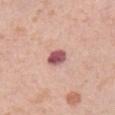Clinical impression:
Captured during whole-body skin photography for melanoma surveillance; the lesion was not biopsied.
Acquisition and patient details:
The tile uses white-light illumination. A 15 mm close-up extracted from a 3D total-body photography capture. A female patient, aged around 40. Located on the left thigh. About 2.5 mm across.